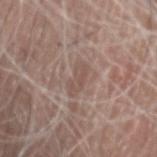{"image": {"source": "total-body photography crop", "field_of_view_mm": 15}, "lighting": "white-light", "site": "head or neck", "automated_metrics": {"area_mm2_approx": 5.5, "eccentricity": 0.9, "shape_asymmetry": 0.25, "border_irregularity_0_10": 3.0, "color_variation_0_10": 2.0, "peripheral_color_asymmetry": 0.5, "nevus_likeness_0_100": 0, "lesion_detection_confidence_0_100": 55}, "patient": {"sex": "male", "age_approx": 80}, "lesion_size": {"long_diameter_mm_approx": 3.5}}